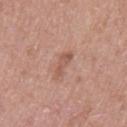Findings:
* biopsy status · imaged on a skin check; not biopsied
* acquisition · ~15 mm tile from a whole-body skin photo
* subject · female, aged approximately 50
* diameter · about 3 mm
* body site · the left upper arm